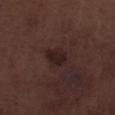Impression: Imaged during a routine full-body skin examination; the lesion was not biopsied and no histopathology is available. Acquisition and patient details: Automated image analysis of the tile measured a lesion area of about 5.5 mm², an eccentricity of roughly 0.55, and a symmetry-axis asymmetry near 0.25. The analysis additionally found a border-irregularity index near 2/10 and a peripheral color-asymmetry measure near 0.5. It also reported a classifier nevus-likeness of about 75/100 and a lesion-detection confidence of about 100/100. Cropped from a total-body skin-imaging series; the visible field is about 15 mm. The patient is a male aged 68 to 72. Longest diameter approximately 3 mm. The lesion is on the left lower leg.